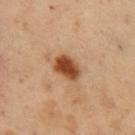Q: Is there a histopathology result?
A: catalogued during a skin exam; not biopsied
Q: What are the patient's age and sex?
A: male, aged approximately 50
Q: Where on the body is the lesion?
A: the chest
Q: What did automated image analysis measure?
A: a shape eccentricity near 0.7 and two-axis asymmetry of about 0.15; a lesion-to-skin contrast of about 11.5 (normalized; higher = more distinct); a classifier nevus-likeness of about 100/100
Q: What kind of image is this?
A: 15 mm crop, total-body photography